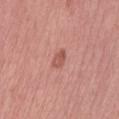biopsy_status: not biopsied; imaged during a skin examination
image:
  source: total-body photography crop
  field_of_view_mm: 15
patient:
  sex: female
  age_approx: 55
site: left thigh
lighting: white-light